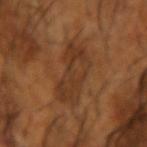The lesion was photographed on a routine skin check and not biopsied; there is no pathology result.
A male patient approximately 65 years of age.
A region of skin cropped from a whole-body photographic capture, roughly 15 mm wide.
Located on the arm.